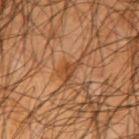  biopsy_status: not biopsied; imaged during a skin examination
  lesion_size:
    long_diameter_mm_approx: 2.5
  site: right upper arm
  image:
    source: total-body photography crop
    field_of_view_mm: 15
  lighting: cross-polarized
  automated_metrics:
    area_mm2_approx: 2.5
    eccentricity: 0.75
    shape_asymmetry: 0.45
    vs_skin_darker_L: 8.0
  patient:
    sex: male
    age_approx: 45